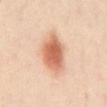Findings:
- workup · catalogued during a skin exam; not biopsied
- illumination · cross-polarized
- body site · the abdomen
- subject · male, aged 48–52
- image · 15 mm crop, total-body photography
- image-analysis metrics · an average lesion color of about L≈64 a*≈25 b*≈34 (CIELAB), roughly 15 lightness units darker than nearby skin, and a normalized border contrast of about 9.5; a nevus-likeness score of about 100/100 and lesion-presence confidence of about 100/100